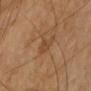biopsy status = no biopsy performed (imaged during a skin exam); subject = male, aged 58 to 62; imaging modality = ~15 mm tile from a whole-body skin photo; lesion diameter = ≈2.5 mm; anatomic site = the arm; tile lighting = cross-polarized illumination.